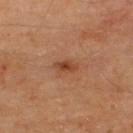  biopsy_status: not biopsied; imaged during a skin examination
  automated_metrics:
    area_mm2_approx: 3.0
    vs_skin_darker_L: 10.0
    vs_skin_contrast_norm: 8.0
    nevus_likeness_0_100: 60
    lesion_detection_confidence_0_100: 100
  lesion_size:
    long_diameter_mm_approx: 2.5
  patient:
    sex: male
    age_approx: 65
  site: upper back
  image:
    source: total-body photography crop
    field_of_view_mm: 15